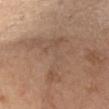<record>
<biopsy_status>not biopsied; imaged during a skin examination</biopsy_status>
<site>chest</site>
<patient>
  <sex>female</sex>
  <age_approx>70</age_approx>
</patient>
<lighting>white-light</lighting>
<automated_metrics>
  <border_irregularity_0_10>9.5</border_irregularity_0_10>
  <color_variation_0_10>3.5</color_variation_0_10>
  <peripheral_color_asymmetry>1.0</peripheral_color_asymmetry>
  <lesion_detection_confidence_0_100>70</lesion_detection_confidence_0_100>
</automated_metrics>
<image>
  <source>total-body photography crop</source>
  <field_of_view_mm>15</field_of_view_mm>
</image>
<lesion_size>
  <long_diameter_mm_approx>7.5</long_diameter_mm_approx>
</lesion_size>
</record>